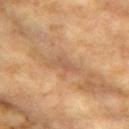Captured during whole-body skin photography for melanoma surveillance; the lesion was not biopsied. The lesion is located on the left upper arm. Cropped from a whole-body photographic skin survey; the tile spans about 15 mm. The tile uses cross-polarized illumination. A female subject, approximately 75 years of age. Measured at roughly 3 mm in maximum diameter.